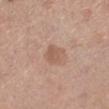This lesion was catalogued during total-body skin photography and was not selected for biopsy.
Automated tile analysis of the lesion measured a normalized border contrast of about 5.5. The software also gave a nevus-likeness score of about 15/100 and a lesion-detection confidence of about 100/100.
Imaged with white-light lighting.
Cropped from a whole-body photographic skin survey; the tile spans about 15 mm.
Measured at roughly 3 mm in maximum diameter.
A female subject aged 53–57.
The lesion is located on the left lower leg.